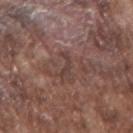<record>
<biopsy_status>not biopsied; imaged during a skin examination</biopsy_status>
<site>right upper arm</site>
<patient>
  <sex>male</sex>
  <age_approx>75</age_approx>
</patient>
<image>
  <source>total-body photography crop</source>
  <field_of_view_mm>15</field_of_view_mm>
</image>
</record>